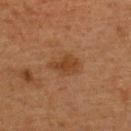| field | value |
|---|---|
| workup | catalogued during a skin exam; not biopsied |
| patient | female, in their 50s |
| lighting | cross-polarized illumination |
| image-analysis metrics | roughly 7 lightness units darker than nearby skin and a lesion-to-skin contrast of about 7 (normalized; higher = more distinct); a border-irregularity index near 3/10, a color-variation rating of about 2/10, and radial color variation of about 0.5; an automated nevus-likeness rating near 70 out of 100 and lesion-presence confidence of about 100/100 |
| body site | the back |
| image source | ~15 mm crop, total-body skin-cancer survey |
| lesion diameter | ~3.5 mm (longest diameter) |